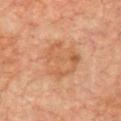• follow-up: imaged on a skin check; not biopsied
• lighting: cross-polarized illumination
• subject: male, aged around 75
• imaging modality: ~15 mm crop, total-body skin-cancer survey
• site: the front of the torso
• diameter: about 5 mm
• automated lesion analysis: a lesion color around L≈49 a*≈19 b*≈31 in CIELAB and a lesion-to-skin contrast of about 5 (normalized; higher = more distinct); lesion-presence confidence of about 100/100